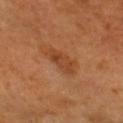notes = imaged on a skin check; not biopsied
subject = male, aged approximately 60
anatomic site = the head or neck
image source = 15 mm crop, total-body photography
tile lighting = cross-polarized illumination
lesion diameter = about 3.5 mm